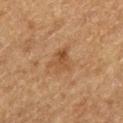Case summary:
• follow-up — catalogued during a skin exam; not biopsied
• patient — male, aged around 75
• body site — the left thigh
• image source — ~15 mm crop, total-body skin-cancer survey